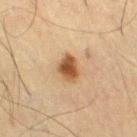Captured during whole-body skin photography for melanoma surveillance; the lesion was not biopsied. A close-up tile cropped from a whole-body skin photograph, about 15 mm across. The subject is a male aged 48 to 52. The recorded lesion diameter is about 3 mm. Captured under cross-polarized illumination. The lesion is located on the right thigh. Automated tile analysis of the lesion measured a lesion–skin lightness drop of about 13 and a normalized border contrast of about 10.5.